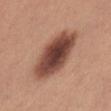biopsy_status: not biopsied; imaged during a skin examination
image:
  source: total-body photography crop
  field_of_view_mm: 15
patient:
  sex: female
  age_approx: 25
site: lower back